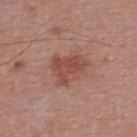Captured during whole-body skin photography for melanoma surveillance; the lesion was not biopsied. About 5 mm across. A 15 mm close-up tile from a total-body photography series done for melanoma screening. The patient is a male aged 38–42. Automated image analysis of the tile measured a footprint of about 12 mm², an eccentricity of roughly 0.7, and a symmetry-axis asymmetry near 0.3. The software also gave a lesion color around L≈49 a*≈24 b*≈26 in CIELAB, roughly 9 lightness units darker than nearby skin, and a normalized border contrast of about 6.5. The software also gave internal color variation of about 3 on a 0–10 scale and radial color variation of about 1. The lesion is located on the upper back. Captured under white-light illumination.